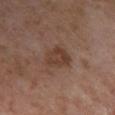lesion diameter: ~3 mm (longest diameter)
location: the right lower leg
automated lesion analysis: a footprint of about 7 mm², an outline eccentricity of about 0.4 (0 = round, 1 = elongated), and a shape-asymmetry score of about 0.25 (0 = symmetric); a lesion color around L≈39 a*≈18 b*≈26 in CIELAB, a lesion–skin lightness drop of about 8, and a lesion-to-skin contrast of about 7 (normalized; higher = more distinct); a nevus-likeness score of about 5/100 and a detector confidence of about 100 out of 100 that the crop contains a lesion
illumination: cross-polarized illumination
patient: female, about 55 years old
imaging modality: ~15 mm tile from a whole-body skin photo